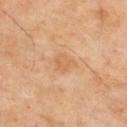Case summary:
– biopsy status — imaged on a skin check; not biopsied
– body site — the mid back
– patient — male, aged approximately 55
– acquisition — ~15 mm crop, total-body skin-cancer survey
– lighting — cross-polarized illumination